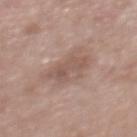Clinical summary:
The lesion is on the upper back. The recorded lesion diameter is about 4 mm. A close-up tile cropped from a whole-body skin photograph, about 15 mm across. The total-body-photography lesion software estimated a mean CIELAB color near L≈53 a*≈17 b*≈24. Captured under white-light illumination. A female patient aged 48–52.